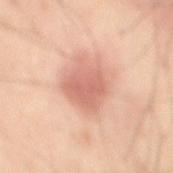notes=total-body-photography surveillance lesion; no biopsy
tile lighting=cross-polarized
subject=roughly 55 years of age
image=15 mm crop, total-body photography
body site=the back
lesion size=~4.5 mm (longest diameter)
automated metrics=a border-irregularity rating of about 3/10 and peripheral color asymmetry of about 1; an automated nevus-likeness rating near 85 out of 100 and lesion-presence confidence of about 100/100